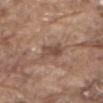Clinical impression:
Captured during whole-body skin photography for melanoma surveillance; the lesion was not biopsied.
Clinical summary:
Located on the abdomen. A male patient aged around 80. A roughly 15 mm field-of-view crop from a total-body skin photograph.